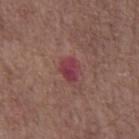Part of a total-body skin-imaging series; this lesion was reviewed on a skin check and was not flagged for biopsy.
The lesion is on the front of the torso.
Approximately 2.5 mm at its widest.
A male subject, in their 70s.
The total-body-photography lesion software estimated an area of roughly 5 mm², an eccentricity of roughly 0.55, and a symmetry-axis asymmetry near 0.25. And it measured a color-variation rating of about 3.5/10 and a peripheral color-asymmetry measure near 1.5. The analysis additionally found a lesion-detection confidence of about 100/100.
A 15 mm crop from a total-body photograph taken for skin-cancer surveillance.
Captured under white-light illumination.